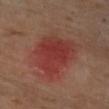Recorded during total-body skin imaging; not selected for excision or biopsy.
A female patient aged 53 to 57.
The lesion is on the arm.
Imaged with cross-polarized lighting.
A 15 mm crop from a total-body photograph taken for skin-cancer surveillance.
Approximately 6 mm at its widest.